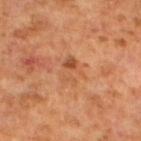Q: Was this lesion biopsied?
A: total-body-photography surveillance lesion; no biopsy